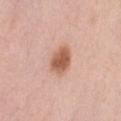This lesion was catalogued during total-body skin photography and was not selected for biopsy.
Located on the left thigh.
A female subject aged approximately 50.
A lesion tile, about 15 mm wide, cut from a 3D total-body photograph.